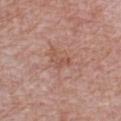No biopsy was performed on this lesion — it was imaged during a full skin examination and was not determined to be concerning.
The lesion is located on the front of the torso.
This is a white-light tile.
A lesion tile, about 15 mm wide, cut from a 3D total-body photograph.
The subject is a male in their 50s.
Longest diameter approximately 2.5 mm.